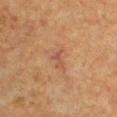Q: Is there a histopathology result?
A: no biopsy performed (imaged during a skin exam)
Q: What is the imaging modality?
A: ~15 mm tile from a whole-body skin photo
Q: Where on the body is the lesion?
A: the chest
Q: Automated lesion metrics?
A: a border-irregularity index near 6.5/10, a within-lesion color-variation index near 0/10, and a peripheral color-asymmetry measure near 0
Q: Illumination type?
A: cross-polarized illumination
Q: What are the patient's age and sex?
A: male, in their 50s
Q: Lesion size?
A: about 2.5 mm